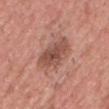Part of a total-body skin-imaging series; this lesion was reviewed on a skin check and was not flagged for biopsy. A male subject, about 40 years old. From the head or neck. This image is a 15 mm lesion crop taken from a total-body photograph.